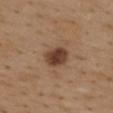Clinical impression:
Part of a total-body skin-imaging series; this lesion was reviewed on a skin check and was not flagged for biopsy.
Clinical summary:
The lesion is on the upper back. Imaged with white-light lighting. A close-up tile cropped from a whole-body skin photograph, about 15 mm across. A female subject about 40 years old.